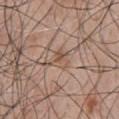No biopsy was performed on this lesion — it was imaged during a full skin examination and was not determined to be concerning. A 15 mm close-up tile from a total-body photography series done for melanoma screening. About 3.5 mm across. The lesion is located on the chest. A male subject, aged 48 to 52. The total-body-photography lesion software estimated an automated nevus-likeness rating near 0 out of 100 and a lesion-detection confidence of about 90/100.